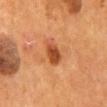{
  "lighting": "cross-polarized",
  "site": "mid back",
  "image": {
    "source": "total-body photography crop",
    "field_of_view_mm": 15
  },
  "patient": {
    "sex": "male",
    "age_approx": 55
  },
  "lesion_size": {
    "long_diameter_mm_approx": 3.5
  }
}